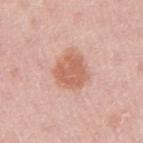Q: Was this lesion biopsied?
A: imaged on a skin check; not biopsied
Q: Lesion size?
A: ≈4 mm
Q: Patient demographics?
A: female, aged approximately 30
Q: Where on the body is the lesion?
A: the right upper arm
Q: How was this image acquired?
A: ~15 mm tile from a whole-body skin photo